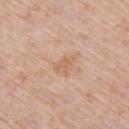size = about 2.5 mm | lighting = white-light | subject = male, aged around 50 | acquisition = ~15 mm tile from a whole-body skin photo | body site = the upper back.